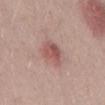Findings:
– follow-up · catalogued during a skin exam; not biopsied
– image · ~15 mm crop, total-body skin-cancer survey
– diameter · ~3.5 mm (longest diameter)
– automated lesion analysis · an area of roughly 7.5 mm²; an automated nevus-likeness rating near 30 out of 100
– subject · male, in their 40s
– illumination · white-light
– site · the back